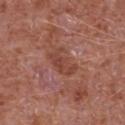* notes: imaged on a skin check; not biopsied
* TBP lesion metrics: an eccentricity of roughly 0.8 and two-axis asymmetry of about 0.35; roughly 7 lightness units darker than nearby skin and a lesion-to-skin contrast of about 6 (normalized; higher = more distinct); a nevus-likeness score of about 0/100 and a lesion-detection confidence of about 100/100
* lighting: white-light illumination
* anatomic site: the chest
* acquisition: ~15 mm tile from a whole-body skin photo
* patient: male, aged 63–67
* size: ~4 mm (longest diameter)